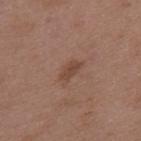Clinical impression:
Part of a total-body skin-imaging series; this lesion was reviewed on a skin check and was not flagged for biopsy.
Context:
The tile uses white-light illumination. About 3 mm across. From the upper back. A male patient aged 48 to 52. An algorithmic analysis of the crop reported a border-irregularity rating of about 3/10. A region of skin cropped from a whole-body photographic capture, roughly 15 mm wide.